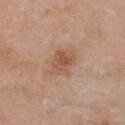Notes:
* follow-up: no biopsy performed (imaged during a skin exam)
* lighting: white-light
* location: the front of the torso
* acquisition: total-body-photography crop, ~15 mm field of view
* patient: female, roughly 40 years of age